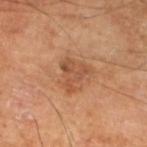<tbp_lesion>
  <biopsy_status>not biopsied; imaged during a skin examination</biopsy_status>
  <lighting>cross-polarized</lighting>
  <patient>
    <sex>male</sex>
    <age_approx>65</age_approx>
  </patient>
  <lesion_size>
    <long_diameter_mm_approx>3.5</long_diameter_mm_approx>
  </lesion_size>
  <image>
    <source>total-body photography crop</source>
    <field_of_view_mm>15</field_of_view_mm>
  </image>
  <site>left lower leg</site>
</tbp_lesion>